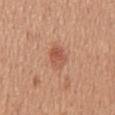No biopsy was performed on this lesion — it was imaged during a full skin examination and was not determined to be concerning.
An algorithmic analysis of the crop reported a border-irregularity index near 2/10 and peripheral color asymmetry of about 1.5. The software also gave an automated nevus-likeness rating near 85 out of 100.
A lesion tile, about 15 mm wide, cut from a 3D total-body photograph.
The lesion's longest dimension is about 3 mm.
Imaged with white-light lighting.
Located on the back.
The subject is a female aged around 55.